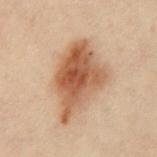Clinical impression:
Part of a total-body skin-imaging series; this lesion was reviewed on a skin check and was not flagged for biopsy.
Acquisition and patient details:
The lesion is located on the chest. A lesion tile, about 15 mm wide, cut from a 3D total-body photograph. A female patient approximately 30 years of age. This is a cross-polarized tile. The recorded lesion diameter is about 7.5 mm.